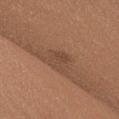Clinical impression: The lesion was photographed on a routine skin check and not biopsied; there is no pathology result. Clinical summary: A region of skin cropped from a whole-body photographic capture, roughly 15 mm wide. The lesion-visualizer software estimated an average lesion color of about L≈45 a*≈19 b*≈29 (CIELAB), about 6 CIELAB-L* units darker than the surrounding skin, and a lesion-to-skin contrast of about 5 (normalized; higher = more distinct). The software also gave border irregularity of about 2.5 on a 0–10 scale, a within-lesion color-variation index near 3/10, and radial color variation of about 1. Imaged with white-light lighting. The lesion is on the chest. The patient is a male aged approximately 25. Measured at roughly 2.5 mm in maximum diameter.